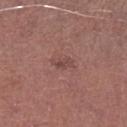Recorded during total-body skin imaging; not selected for excision or biopsy. Approximately 2.5 mm at its widest. A 15 mm crop from a total-body photograph taken for skin-cancer surveillance. Automated image analysis of the tile measured a lesion area of about 3.5 mm², a shape eccentricity near 0.85, and two-axis asymmetry of about 0.35. It also reported internal color variation of about 2.5 on a 0–10 scale and peripheral color asymmetry of about 1. It also reported a classifier nevus-likeness of about 5/100 and lesion-presence confidence of about 100/100. The tile uses white-light illumination. A male patient, in their mid-60s. The lesion is located on the left lower leg.